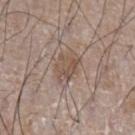workup = catalogued during a skin exam; not biopsied